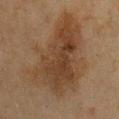Q: Was a biopsy performed?
A: no biopsy performed (imaged during a skin exam)
Q: What kind of image is this?
A: ~15 mm tile from a whole-body skin photo
Q: Illumination type?
A: cross-polarized illumination
Q: What is the lesion's diameter?
A: ~10.5 mm (longest diameter)
Q: Where on the body is the lesion?
A: the chest
Q: Patient demographics?
A: female, aged 53–57
Q: What did automated image analysis measure?
A: a lesion area of about 50 mm², an eccentricity of roughly 0.75, and a symmetry-axis asymmetry near 0.35; a mean CIELAB color near L≈35 a*≈15 b*≈27, roughly 7 lightness units darker than nearby skin, and a lesion-to-skin contrast of about 7.5 (normalized; higher = more distinct); radial color variation of about 1.5